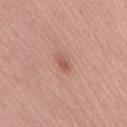The lesion was tiled from a total-body skin photograph and was not biopsied. Located on the right thigh. A 15 mm close-up extracted from a 3D total-body photography capture. A female patient in their mid- to late 40s. Longest diameter approximately 2.5 mm. The tile uses white-light illumination.